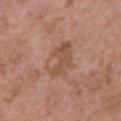Notes:
• body site — the chest
• tile lighting — white-light
• diameter — ~5 mm (longest diameter)
• image source — ~15 mm tile from a whole-body skin photo
• subject — female, aged 38 to 42